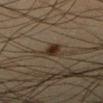The lesion was photographed on a routine skin check and not biopsied; there is no pathology result.
A male patient in their mid-30s.
Measured at roughly 4 mm in maximum diameter.
A lesion tile, about 15 mm wide, cut from a 3D total-body photograph.
Imaged with cross-polarized lighting.
From the right lower leg.